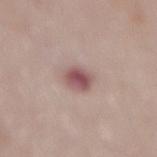notes: no biopsy performed (imaged during a skin exam) | patient: female, aged 68 to 72 | automated lesion analysis: border irregularity of about 1.5 on a 0–10 scale, a within-lesion color-variation index near 4.5/10, and a peripheral color-asymmetry measure near 1; a nevus-likeness score of about 0/100 | site: the mid back | image: ~15 mm crop, total-body skin-cancer survey.